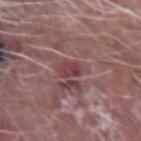Impression: No biopsy was performed on this lesion — it was imaged during a full skin examination and was not determined to be concerning. Clinical summary: On the right forearm. This is a white-light tile. The subject is a male aged 63–67. The lesion's longest dimension is about 3 mm. Cropped from a whole-body photographic skin survey; the tile spans about 15 mm.